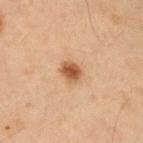Impression: Recorded during total-body skin imaging; not selected for excision or biopsy. Context: A male patient, roughly 50 years of age. A region of skin cropped from a whole-body photographic capture, roughly 15 mm wide. From the right upper arm. The total-body-photography lesion software estimated a lesion area of about 4.5 mm² and an outline eccentricity of about 0.6 (0 = round, 1 = elongated). And it measured a lesion color around L≈42 a*≈18 b*≈30 in CIELAB, a lesion–skin lightness drop of about 11, and a lesion-to-skin contrast of about 9.5 (normalized; higher = more distinct). The software also gave a peripheral color-asymmetry measure near 0.5. The software also gave a lesion-detection confidence of about 100/100. This is a cross-polarized tile.